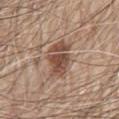biopsy status: total-body-photography surveillance lesion; no biopsy | imaging modality: ~15 mm crop, total-body skin-cancer survey | tile lighting: white-light illumination | subject: male, in their 80s | site: the abdomen | automated lesion analysis: a lesion area of about 12 mm² and two-axis asymmetry of about 0.25; an average lesion color of about L≈49 a*≈19 b*≈27 (CIELAB), about 12 CIELAB-L* units darker than the surrounding skin, and a lesion-to-skin contrast of about 9 (normalized; higher = more distinct); a border-irregularity rating of about 3/10, a color-variation rating of about 4.5/10, and a peripheral color-asymmetry measure near 1.5; lesion-presence confidence of about 100/100 | diameter: about 5 mm.